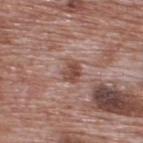| key | value |
|---|---|
| notes | no biopsy performed (imaged during a skin exam) |
| illumination | white-light illumination |
| diameter | ≈2.5 mm |
| subject | male, aged 68 to 72 |
| site | the upper back |
| acquisition | ~15 mm crop, total-body skin-cancer survey |